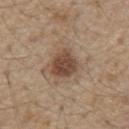The lesion is located on the chest. About 3.5 mm across. A male subject aged 68–72. A lesion tile, about 15 mm wide, cut from a 3D total-body photograph.